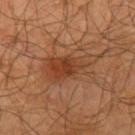Imaged during a routine full-body skin examination; the lesion was not biopsied and no histopathology is available.